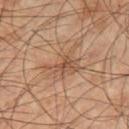| feature | finding |
|---|---|
| notes | imaged on a skin check; not biopsied |